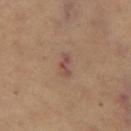This is a cross-polarized tile. About 3 mm across. A female patient aged 68 to 72. Cropped from a total-body skin-imaging series; the visible field is about 15 mm. The lesion is located on the left thigh.